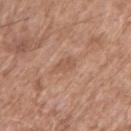{
  "biopsy_status": "not biopsied; imaged during a skin examination",
  "lesion_size": {
    "long_diameter_mm_approx": 2.5
  },
  "image": {
    "source": "total-body photography crop",
    "field_of_view_mm": 15
  },
  "lighting": "white-light",
  "patient": {
    "sex": "male",
    "age_approx": 75
  },
  "site": "right upper arm",
  "automated_metrics": {
    "area_mm2_approx": 4.0,
    "eccentricity": 0.7,
    "shape_asymmetry": 0.2,
    "cielab_L": 55,
    "cielab_a": 20,
    "cielab_b": 30
  }
}